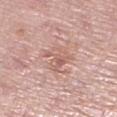Q: Was a biopsy performed?
A: total-body-photography surveillance lesion; no biopsy
Q: What kind of image is this?
A: 15 mm crop, total-body photography
Q: What are the patient's age and sex?
A: female, roughly 60 years of age
Q: What is the anatomic site?
A: the right lower leg